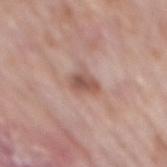<tbp_lesion>
<biopsy_status>not biopsied; imaged during a skin examination</biopsy_status>
<automated_metrics>
  <vs_skin_darker_L>11.0</vs_skin_darker_L>
  <vs_skin_contrast_norm>7.5</vs_skin_contrast_norm>
  <border_irregularity_0_10>2.5</border_irregularity_0_10>
  <color_variation_0_10>3.5</color_variation_0_10>
  <peripheral_color_asymmetry>1.0</peripheral_color_asymmetry>
</automated_metrics>
<image>
  <source>total-body photography crop</source>
  <field_of_view_mm>15</field_of_view_mm>
</image>
<site>mid back</site>
<patient>
  <sex>male</sex>
  <age_approx>75</age_approx>
</patient>
</tbp_lesion>